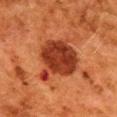Captured during whole-body skin photography for melanoma surveillance; the lesion was not biopsied.
A region of skin cropped from a whole-body photographic capture, roughly 15 mm wide.
Approximately 6 mm at its widest.
The subject is a female aged around 50.
Located on the upper back.
Imaged with cross-polarized lighting.